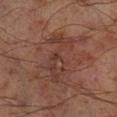Impression: Recorded during total-body skin imaging; not selected for excision or biopsy. Image and clinical context: A male subject, about 70 years old. The recorded lesion diameter is about 6.5 mm. The tile uses cross-polarized illumination. A region of skin cropped from a whole-body photographic capture, roughly 15 mm wide. On the right lower leg. An algorithmic analysis of the crop reported an area of roughly 13 mm² and an eccentricity of roughly 0.9. And it measured border irregularity of about 9 on a 0–10 scale, internal color variation of about 4 on a 0–10 scale, and a peripheral color-asymmetry measure near 1.5. And it measured an automated nevus-likeness rating near 0 out of 100 and lesion-presence confidence of about 85/100.